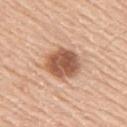location: the right upper arm | imaging modality: ~15 mm crop, total-body skin-cancer survey | diameter: about 4.5 mm | patient: male, aged approximately 60.